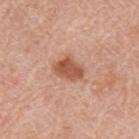The tile uses white-light illumination.
A lesion tile, about 15 mm wide, cut from a 3D total-body photograph.
A male subject, roughly 80 years of age.
On the left upper arm.